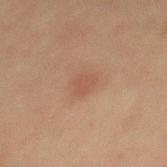Assessment:
Imaged during a routine full-body skin examination; the lesion was not biopsied and no histopathology is available.
Image and clinical context:
This image is a 15 mm lesion crop taken from a total-body photograph. The lesion's longest dimension is about 2.5 mm. The subject is a male roughly 60 years of age. Automated image analysis of the tile measured a footprint of about 4.5 mm², a shape eccentricity near 0.6, and a shape-asymmetry score of about 0.25 (0 = symmetric). The lesion is located on the mid back.